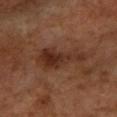This is a cross-polarized tile. Located on the left forearm. A female patient, about 70 years old. This image is a 15 mm lesion crop taken from a total-body photograph.